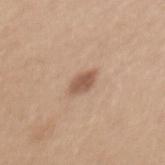Q: Is there a histopathology result?
A: imaged on a skin check; not biopsied
Q: Where on the body is the lesion?
A: the mid back
Q: What did automated image analysis measure?
A: two-axis asymmetry of about 0.2; an automated nevus-likeness rating near 85 out of 100 and a lesion-detection confidence of about 100/100
Q: How was this image acquired?
A: ~15 mm crop, total-body skin-cancer survey
Q: How was the tile lit?
A: white-light illumination
Q: What is the lesion's diameter?
A: ~2.5 mm (longest diameter)
Q: What are the patient's age and sex?
A: female, about 55 years old